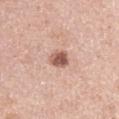The lesion was tiled from a total-body skin photograph and was not biopsied.
Imaged with white-light lighting.
About 2.5 mm across.
A female patient, roughly 50 years of age.
A 15 mm crop from a total-body photograph taken for skin-cancer surveillance.
Automated image analysis of the tile measured a lesion color around L≈57 a*≈22 b*≈27 in CIELAB and a normalized lesion–skin contrast near 9.5. The software also gave an automated nevus-likeness rating near 90 out of 100 and lesion-presence confidence of about 100/100.
Located on the left upper arm.